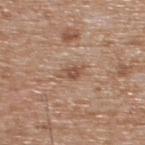Recorded during total-body skin imaging; not selected for excision or biopsy. The tile uses white-light illumination. The lesion is on the upper back. A male patient about 65 years old. A lesion tile, about 15 mm wide, cut from a 3D total-body photograph.